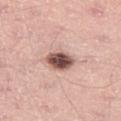Case summary:
- follow-up · no biopsy performed (imaged during a skin exam)
- body site · the left thigh
- imaging modality · ~15 mm crop, total-body skin-cancer survey
- lesion size · ≈4 mm
- subject · male, aged around 60
- automated metrics · a lesion area of about 7.5 mm², an eccentricity of roughly 0.75, and two-axis asymmetry of about 0.1; an average lesion color of about L≈52 a*≈20 b*≈23 (CIELAB), roughly 21 lightness units darker than nearby skin, and a lesion-to-skin contrast of about 13 (normalized; higher = more distinct); border irregularity of about 1 on a 0–10 scale, a within-lesion color-variation index near 6/10, and peripheral color asymmetry of about 2
- illumination · white-light illumination